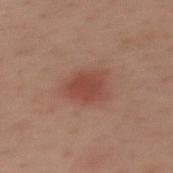{"biopsy_status": "not biopsied; imaged during a skin examination", "site": "mid back", "image": {"source": "total-body photography crop", "field_of_view_mm": 15}, "lighting": "cross-polarized", "lesion_size": {"long_diameter_mm_approx": 3.0}, "patient": {"sex": "male", "age_approx": 40}}